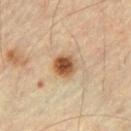biopsy_status: not biopsied; imaged during a skin examination
lighting: cross-polarized
automated_metrics:
  area_mm2_approx: 7.0
  eccentricity: 0.45
  shape_asymmetry: 0.15
  cielab_L: 55
  cielab_a: 20
  cielab_b: 36
  vs_skin_darker_L: 16.0
  border_irregularity_0_10: 1.5
  peripheral_color_asymmetry: 2.0
patient:
  sex: male
  age_approx: 55
image:
  source: total-body photography crop
  field_of_view_mm: 15
site: left thigh